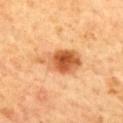Imaged during a routine full-body skin examination; the lesion was not biopsied and no histopathology is available.
Measured at roughly 6 mm in maximum diameter.
The patient is a male in their 60s.
Automated tile analysis of the lesion measured a border-irregularity index near 3/10, internal color variation of about 9.5 on a 0–10 scale, and peripheral color asymmetry of about 3.5.
This is a cross-polarized tile.
Located on the mid back.
Cropped from a total-body skin-imaging series; the visible field is about 15 mm.